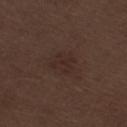notes: no biopsy performed (imaged during a skin exam)
imaging modality: total-body-photography crop, ~15 mm field of view
location: the right thigh
automated lesion analysis: a footprint of about 6 mm² and a shape eccentricity near 0.25; an average lesion color of about L≈26 a*≈15 b*≈19 (CIELAB), roughly 4 lightness units darker than nearby skin, and a lesion-to-skin contrast of about 5 (normalized; higher = more distinct)
diameter: ≈3 mm
patient: male, aged around 70
tile lighting: white-light illumination